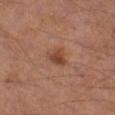This lesion was catalogued during total-body skin photography and was not selected for biopsy. On the leg. The recorded lesion diameter is about 3 mm. This image is a 15 mm lesion crop taken from a total-body photograph. A male subject roughly 55 years of age.